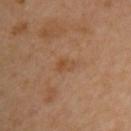<tbp_lesion>
  <biopsy_status>not biopsied; imaged during a skin examination</biopsy_status>
  <image>
    <source>total-body photography crop</source>
    <field_of_view_mm>15</field_of_view_mm>
  </image>
  <automated_metrics>
    <nevus_likeness_0_100>0</nevus_likeness_0_100>
    <lesion_detection_confidence_0_100>100</lesion_detection_confidence_0_100>
  </automated_metrics>
  <lesion_size>
    <long_diameter_mm_approx>2.5</long_diameter_mm_approx>
  </lesion_size>
  <patient>
    <sex>male</sex>
    <age_approx>50</age_approx>
  </patient>
  <lighting>cross-polarized</lighting>
  <site>chest</site>
</tbp_lesion>